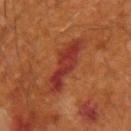No biopsy was performed on this lesion — it was imaged during a full skin examination and was not determined to be concerning.